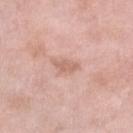This lesion was catalogued during total-body skin photography and was not selected for biopsy. A 15 mm crop from a total-body photograph taken for skin-cancer surveillance. A female patient aged 68 to 72. The tile uses white-light illumination. The recorded lesion diameter is about 3 mm. The lesion is on the right lower leg. Automated tile analysis of the lesion measured a within-lesion color-variation index near 1/10. The software also gave a classifier nevus-likeness of about 0/100 and a detector confidence of about 100 out of 100 that the crop contains a lesion.